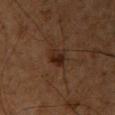biopsy status — imaged on a skin check; not biopsied
patient — male, about 60 years old
image — total-body-photography crop, ~15 mm field of view
site — the left upper arm